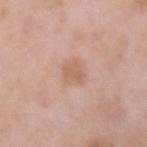{"biopsy_status": "not biopsied; imaged during a skin examination", "lesion_size": {"long_diameter_mm_approx": 2.5}, "patient": {"sex": "female", "age_approx": 65}, "image": {"source": "total-body photography crop", "field_of_view_mm": 15}, "site": "right forearm"}